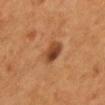Case summary:
- workup: imaged on a skin check; not biopsied
- image-analysis metrics: an area of roughly 5.5 mm², an outline eccentricity of about 0.6 (0 = round, 1 = elongated), and a shape-asymmetry score of about 0.2 (0 = symmetric); a mean CIELAB color near L≈40 a*≈23 b*≈33, about 12 CIELAB-L* units darker than the surrounding skin, and a normalized border contrast of about 9.5; internal color variation of about 5 on a 0–10 scale; a classifier nevus-likeness of about 95/100 and lesion-presence confidence of about 100/100
- image source: total-body-photography crop, ~15 mm field of view
- anatomic site: the mid back
- size: ≈3 mm
- subject: male, aged 53–57
- tile lighting: cross-polarized